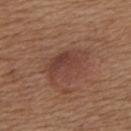notes = imaged on a skin check; not biopsied | diameter = ~5.5 mm (longest diameter) | illumination = white-light illumination | TBP lesion metrics = an eccentricity of roughly 0.75 and two-axis asymmetry of about 0.25 | patient = female, aged 53 to 57 | site = the upper back | imaging modality = ~15 mm tile from a whole-body skin photo.